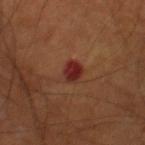Impression: Captured during whole-body skin photography for melanoma surveillance; the lesion was not biopsied. Background: Cropped from a total-body skin-imaging series; the visible field is about 15 mm. From the right thigh. Automated image analysis of the tile measured a border-irregularity index near 1/10, internal color variation of about 3 on a 0–10 scale, and radial color variation of about 1. A male patient aged approximately 70. Longest diameter approximately 2.5 mm.